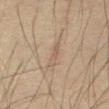workup: no biopsy performed (imaged during a skin exam)
lesion diameter: about 3.5 mm
anatomic site: the front of the torso
acquisition: ~15 mm crop, total-body skin-cancer survey
patient: male, aged approximately 60